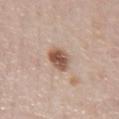notes=catalogued during a skin exam; not biopsied
patient=male, aged 48 to 52
image=~15 mm crop, total-body skin-cancer survey
lesion size=about 3 mm
anatomic site=the chest